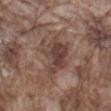Acquisition and patient details:
The lesion-visualizer software estimated a mean CIELAB color near L≈42 a*≈18 b*≈23, about 9 CIELAB-L* units darker than the surrounding skin, and a lesion-to-skin contrast of about 7.5 (normalized; higher = more distinct). The analysis additionally found a color-variation rating of about 4.5/10 and a peripheral color-asymmetry measure near 1.5. The analysis additionally found a nevus-likeness score of about 10/100. A roughly 15 mm field-of-view crop from a total-body skin photograph. On the mid back. This is a white-light tile. Measured at roughly 5.5 mm in maximum diameter. A male patient approximately 75 years of age.